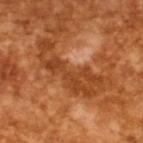The subject is a male in their mid- to late 60s.
A 15 mm crop from a total-body photograph taken for skin-cancer surveillance.